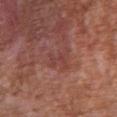{"biopsy_status": "not biopsied; imaged during a skin examination", "image": {"source": "total-body photography crop", "field_of_view_mm": 15}, "site": "chest", "lighting": "white-light", "patient": {"sex": "male", "age_approx": 45}, "lesion_size": {"long_diameter_mm_approx": 2.5}, "automated_metrics": {"border_irregularity_0_10": 6.0, "color_variation_0_10": 0.0, "nevus_likeness_0_100": 0, "lesion_detection_confidence_0_100": 100}}